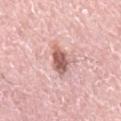workup=imaged on a skin check; not biopsied | tile lighting=white-light illumination | location=the mid back | imaging modality=~15 mm tile from a whole-body skin photo | patient=male, aged 73 to 77.